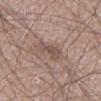Recorded during total-body skin imaging; not selected for excision or biopsy.
The lesion is located on the left thigh.
Automated tile analysis of the lesion measured a color-variation rating of about 1/10 and peripheral color asymmetry of about 0.
Cropped from a total-body skin-imaging series; the visible field is about 15 mm.
A male subject in their mid- to late 60s.